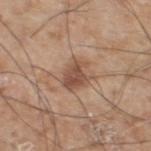{"biopsy_status": "not biopsied; imaged during a skin examination", "lighting": "white-light", "patient": {"sex": "male", "age_approx": 60}, "image": {"source": "total-body photography crop", "field_of_view_mm": 15}, "site": "left lower leg"}